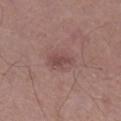Impression:
This lesion was catalogued during total-body skin photography and was not selected for biopsy.
Image and clinical context:
This is a white-light tile. The subject is a male aged 68–72. Automated image analysis of the tile measured a lesion color around L≈46 a*≈21 b*≈21 in CIELAB, a lesion–skin lightness drop of about 8, and a normalized border contrast of about 6.5. The analysis additionally found a within-lesion color-variation index near 1.5/10 and peripheral color asymmetry of about 0.5. And it measured an automated nevus-likeness rating near 15 out of 100 and lesion-presence confidence of about 100/100. A close-up tile cropped from a whole-body skin photograph, about 15 mm across. The lesion is on the right lower leg.